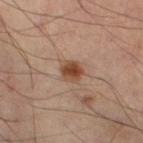A male patient, aged around 50.
Imaged with cross-polarized lighting.
Measured at roughly 3 mm in maximum diameter.
Located on the left leg.
Cropped from a total-body skin-imaging series; the visible field is about 15 mm.
Automated tile analysis of the lesion measured a footprint of about 5.5 mm², an eccentricity of roughly 0.55, and a symmetry-axis asymmetry near 0.2. The software also gave a border-irregularity index near 2/10, a color-variation rating of about 4.5/10, and a peripheral color-asymmetry measure near 1. It also reported a nevus-likeness score of about 95/100.